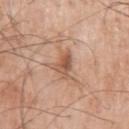Impression: This lesion was catalogued during total-body skin photography and was not selected for biopsy. Clinical summary: A male patient approximately 75 years of age. Automated tile analysis of the lesion measured about 11 CIELAB-L* units darker than the surrounding skin. The software also gave internal color variation of about 4.5 on a 0–10 scale and peripheral color asymmetry of about 1.5. The analysis additionally found an automated nevus-likeness rating near 35 out of 100 and a lesion-detection confidence of about 100/100. Captured under white-light illumination. Located on the left upper arm. The lesion's longest dimension is about 3 mm. A 15 mm close-up extracted from a 3D total-body photography capture.